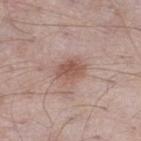| field | value |
|---|---|
| follow-up | imaged on a skin check; not biopsied |
| subject | male, aged 53 to 57 |
| imaging modality | 15 mm crop, total-body photography |
| automated lesion analysis | a lesion area of about 7 mm² and a shape eccentricity near 0.65; a border-irregularity rating of about 2.5/10, a within-lesion color-variation index near 3/10, and peripheral color asymmetry of about 1 |
| body site | the right thigh |
| tile lighting | white-light |